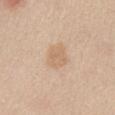No biopsy was performed on this lesion — it was imaged during a full skin examination and was not determined to be concerning. A 15 mm close-up extracted from a 3D total-body photography capture. Located on the chest. Automated image analysis of the tile measured a shape eccentricity near 0.65. And it measured internal color variation of about 2 on a 0–10 scale. The recorded lesion diameter is about 3 mm. The subject is a female about 40 years old. Imaged with white-light lighting.